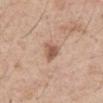Impression:
This lesion was catalogued during total-body skin photography and was not selected for biopsy.
Clinical summary:
A region of skin cropped from a whole-body photographic capture, roughly 15 mm wide. A male subject, in their 60s. Located on the chest.